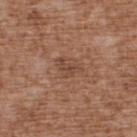follow-up=total-body-photography surveillance lesion; no biopsy
site=the upper back
subject=male, roughly 75 years of age
lighting=white-light illumination
image=~15 mm tile from a whole-body skin photo